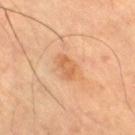Impression:
This lesion was catalogued during total-body skin photography and was not selected for biopsy.
Context:
The tile uses cross-polarized illumination. A subject aged around 65. This image is a 15 mm lesion crop taken from a total-body photograph. Measured at roughly 3 mm in maximum diameter. The lesion is located on the right thigh.